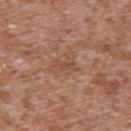imaging modality=total-body-photography crop, ~15 mm field of view
illumination=white-light
patient=male, approximately 45 years of age
automated lesion analysis=a mean CIELAB color near L≈49 a*≈23 b*≈30; a nevus-likeness score of about 0/100 and a lesion-detection confidence of about 100/100
lesion size=about 2.5 mm
location=the upper back